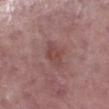Captured during whole-body skin photography for melanoma surveillance; the lesion was not biopsied.
Captured under white-light illumination.
A lesion tile, about 15 mm wide, cut from a 3D total-body photograph.
A male patient, aged 38 to 42.
Longest diameter approximately 3 mm.
On the left lower leg.